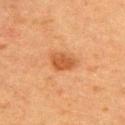Clinical impression: Recorded during total-body skin imaging; not selected for excision or biopsy. Context: The patient is a female aged 53–57. A close-up tile cropped from a whole-body skin photograph, about 15 mm across. From the back.